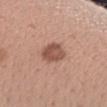This lesion was catalogued during total-body skin photography and was not selected for biopsy.
The tile uses white-light illumination.
About 3 mm across.
The subject is a female in their mid-20s.
Located on the right forearm.
A 15 mm close-up tile from a total-body photography series done for melanoma screening.